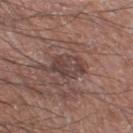Assessment: This lesion was catalogued during total-body skin photography and was not selected for biopsy. Clinical summary: A roughly 15 mm field-of-view crop from a total-body skin photograph. The lesion is located on the left thigh. About 5 mm across. Captured under white-light illumination. A male subject, about 60 years old.